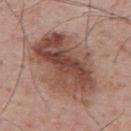<case>
  <biopsy_status>not biopsied; imaged during a skin examination</biopsy_status>
  <image>
    <source>total-body photography crop</source>
    <field_of_view_mm>15</field_of_view_mm>
  </image>
  <site>upper back</site>
  <lighting>white-light</lighting>
  <patient>
    <sex>male</sex>
    <age_approx>55</age_approx>
  </patient>
</case>